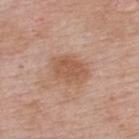Q: Was this lesion biopsied?
A: catalogued during a skin exam; not biopsied
Q: How was this image acquired?
A: 15 mm crop, total-body photography
Q: Patient demographics?
A: male, aged 58 to 62
Q: Where on the body is the lesion?
A: the back